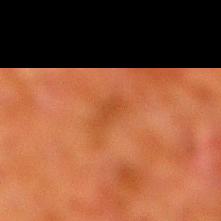notes = no biopsy performed (imaged during a skin exam) | automated lesion analysis = a footprint of about 3 mm², an eccentricity of roughly 0.85, and a symmetry-axis asymmetry near 0.25; a lesion color around L≈38 a*≈26 b*≈36 in CIELAB, about 5 CIELAB-L* units darker than the surrounding skin, and a normalized lesion–skin contrast near 4.5; a border-irregularity rating of about 3/10, internal color variation of about 0.5 on a 0–10 scale, and radial color variation of about 0; an automated nevus-likeness rating near 0 out of 100 and a lesion-detection confidence of about 100/100 | body site = the right lower leg | subject = male, aged around 80 | illumination = cross-polarized | image source = total-body-photography crop, ~15 mm field of view | size = ~2.5 mm (longest diameter).